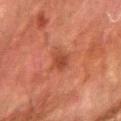Impression:
Recorded during total-body skin imaging; not selected for excision or biopsy.
Context:
The subject is a male aged 78 to 82. A 15 mm close-up extracted from a 3D total-body photography capture. Located on the right forearm. The total-body-photography lesion software estimated a footprint of about 4.5 mm² and a symmetry-axis asymmetry near 0.25. And it measured an average lesion color of about L≈36 a*≈24 b*≈28 (CIELAB). And it measured peripheral color asymmetry of about 0.5. And it measured a nevus-likeness score of about 5/100 and a detector confidence of about 100 out of 100 that the crop contains a lesion.